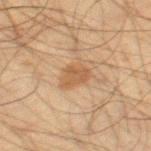automated lesion analysis: a footprint of about 5.5 mm², a shape eccentricity near 0.7, and two-axis asymmetry of about 0.3; a lesion color around L≈47 a*≈17 b*≈31 in CIELAB, a lesion–skin lightness drop of about 8, and a normalized border contrast of about 6.5; a nevus-likeness score of about 20/100 | patient: male, approximately 60 years of age | lighting: cross-polarized illumination | location: the leg | acquisition: 15 mm crop, total-body photography.